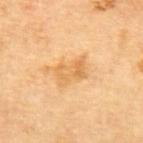Assessment: Imaged during a routine full-body skin examination; the lesion was not biopsied and no histopathology is available. Context: A female subject in their mid-60s. The tile uses cross-polarized illumination. A close-up tile cropped from a whole-body skin photograph, about 15 mm across. From the upper back.